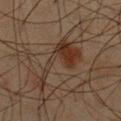Assessment:
Recorded during total-body skin imaging; not selected for excision or biopsy.
Context:
Approximately 8 mm at its widest. A male subject, aged approximately 45. A 15 mm close-up extracted from a 3D total-body photography capture. Automated image analysis of the tile measured border irregularity of about 10 on a 0–10 scale. From the chest. The tile uses cross-polarized illumination.